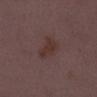* notes: imaged on a skin check; not biopsied
* anatomic site: the right lower leg
* imaging modality: ~15 mm tile from a whole-body skin photo
* automated lesion analysis: an area of roughly 6 mm², a shape eccentricity near 0.75, and two-axis asymmetry of about 0.4; radial color variation of about 0.5
* lesion diameter: ~3 mm (longest diameter)
* subject: female, aged 28–32
* tile lighting: white-light illumination